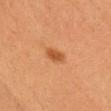<lesion>
<biopsy_status>not biopsied; imaged during a skin examination</biopsy_status>
<lesion_size>
  <long_diameter_mm_approx>2.5</long_diameter_mm_approx>
</lesion_size>
<automated_metrics>
  <eccentricity>0.75</eccentricity>
  <shape_asymmetry>0.2</shape_asymmetry>
  <border_irregularity_0_10>1.5</border_irregularity_0_10>
  <color_variation_0_10>2.0</color_variation_0_10>
  <peripheral_color_asymmetry>0.5</peripheral_color_asymmetry>
  <nevus_likeness_0_100>95</nevus_likeness_0_100>
  <lesion_detection_confidence_0_100>100</lesion_detection_confidence_0_100>
</automated_metrics>
<image>
  <source>total-body photography crop</source>
  <field_of_view_mm>15</field_of_view_mm>
</image>
<patient>
  <sex>female</sex>
  <age_approx>40</age_approx>
</patient>
<lighting>cross-polarized</lighting>
<site>head or neck</site>
</lesion>